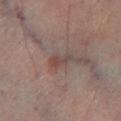Case summary:
* workup: imaged on a skin check; not biopsied
* image source: total-body-photography crop, ~15 mm field of view
* tile lighting: cross-polarized
* anatomic site: the left lower leg
* patient: male, roughly 65 years of age